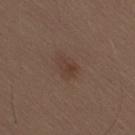Background: Located on the lower back. A region of skin cropped from a whole-body photographic capture, roughly 15 mm wide. The lesion-visualizer software estimated a lesion area of about 4.5 mm², an outline eccentricity of about 0.7 (0 = round, 1 = elongated), and a shape-asymmetry score of about 0.35 (0 = symmetric). The analysis additionally found a lesion color around L≈38 a*≈16 b*≈24 in CIELAB, a lesion–skin lightness drop of about 6, and a lesion-to-skin contrast of about 5.5 (normalized; higher = more distinct). A male subject, about 70 years old. Imaged with white-light lighting.